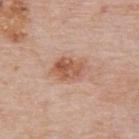Case summary:
– workup: catalogued during a skin exam; not biopsied
– imaging modality: total-body-photography crop, ~15 mm field of view
– automated lesion analysis: a mean CIELAB color near L≈57 a*≈22 b*≈31, about 10 CIELAB-L* units darker than the surrounding skin, and a normalized border contrast of about 7.5; a lesion-detection confidence of about 100/100
– subject: male, approximately 75 years of age
– body site: the back
– lighting: white-light
– size: ~4.5 mm (longest diameter)